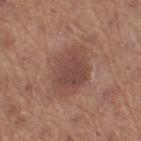Imaged during a routine full-body skin examination; the lesion was not biopsied and no histopathology is available. The lesion is located on the right thigh. Cropped from a whole-body photographic skin survey; the tile spans about 15 mm. A female patient, in their mid-60s.